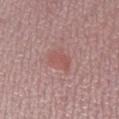Findings:
• biopsy status: imaged on a skin check; not biopsied
• location: the left lower leg
• patient: female, in their mid-50s
• acquisition: total-body-photography crop, ~15 mm field of view